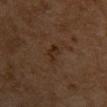- notes · total-body-photography surveillance lesion; no biopsy
- patient · female, roughly 60 years of age
- imaging modality · 15 mm crop, total-body photography
- size · about 3 mm
- lighting · cross-polarized illumination
- anatomic site · the chest